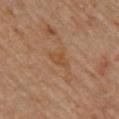biopsy status = total-body-photography surveillance lesion; no biopsy | size = ~3 mm (longest diameter) | subject = male, in their mid- to late 80s or older | site = the upper back | image = 15 mm crop, total-body photography.